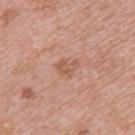From the upper back. A female patient in their 40s. A 15 mm crop from a total-body photograph taken for skin-cancer surveillance.